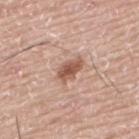  automated_metrics:
    border_irregularity_0_10: 3.0
    color_variation_0_10: 3.0
    peripheral_color_asymmetry: 1.0
  image:
    source: total-body photography crop
    field_of_view_mm: 15
  patient:
    sex: male
    age_approx: 75
  lesion_size:
    long_diameter_mm_approx: 3.5
  lighting: white-light
  site: upper back